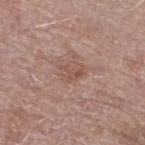The patient is a male aged 73 to 77. Measured at roughly 3 mm in maximum diameter. Captured under white-light illumination. From the right thigh. A close-up tile cropped from a whole-body skin photograph, about 15 mm across.